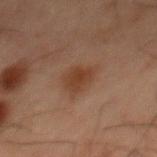Clinical impression: This lesion was catalogued during total-body skin photography and was not selected for biopsy. Image and clinical context: This image is a 15 mm lesion crop taken from a total-body photograph. The lesion is on the mid back. The total-body-photography lesion software estimated a mean CIELAB color near L≈30 a*≈16 b*≈24, a lesion–skin lightness drop of about 6, and a normalized lesion–skin contrast near 7.5. The software also gave a border-irregularity rating of about 2.5/10, internal color variation of about 2 on a 0–10 scale, and radial color variation of about 0.5. It also reported a nevus-likeness score of about 70/100 and a lesion-detection confidence of about 100/100. Imaged with cross-polarized lighting. The patient is a male aged 58–62. Measured at roughly 3.5 mm in maximum diameter.